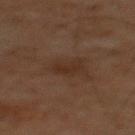biopsy status: catalogued during a skin exam; not biopsied
diameter: ~2.5 mm (longest diameter)
illumination: cross-polarized
acquisition: total-body-photography crop, ~15 mm field of view
subject: male, aged around 60
anatomic site: the chest